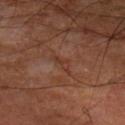This lesion was catalogued during total-body skin photography and was not selected for biopsy.